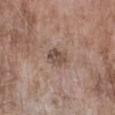{"biopsy_status": "not biopsied; imaged during a skin examination", "lesion_size": {"long_diameter_mm_approx": 3.0}, "patient": {"sex": "female", "age_approx": 75}, "image": {"source": "total-body photography crop", "field_of_view_mm": 15}, "lighting": "white-light", "site": "right forearm"}